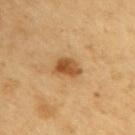Q: Is there a histopathology result?
A: catalogued during a skin exam; not biopsied
Q: How was this image acquired?
A: ~15 mm crop, total-body skin-cancer survey
Q: Where on the body is the lesion?
A: the arm
Q: Who is the patient?
A: male, roughly 60 years of age